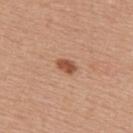{"biopsy_status": "not biopsied; imaged during a skin examination"}